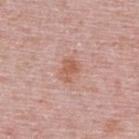No biopsy was performed on this lesion — it was imaged during a full skin examination and was not determined to be concerning.
A male patient, about 50 years old.
A 15 mm close-up tile from a total-body photography series done for melanoma screening.
Captured under white-light illumination.
On the upper back.